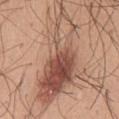Clinical impression: This lesion was catalogued during total-body skin photography and was not selected for biopsy. Acquisition and patient details: Automated image analysis of the tile measured a footprint of about 38 mm² and two-axis asymmetry of about 0.5. The lesion's longest dimension is about 13.5 mm. This is a white-light tile. The lesion is on the chest. A 15 mm close-up tile from a total-body photography series done for melanoma screening. The patient is a male aged around 45.